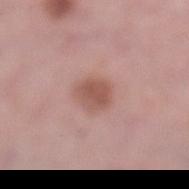Assessment:
Recorded during total-body skin imaging; not selected for excision or biopsy.
Context:
Approximately 3 mm at its widest. Located on the left lower leg. This is a white-light tile. A female subject, aged around 65. Cropped from a whole-body photographic skin survey; the tile spans about 15 mm.